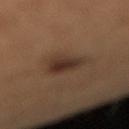From the right lower leg. A 15 mm crop from a total-body photograph taken for skin-cancer surveillance. A female subject, aged 53 to 57.